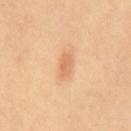Q: Is there a histopathology result?
A: total-body-photography surveillance lesion; no biopsy
Q: What lighting was used for the tile?
A: cross-polarized illumination
Q: What kind of image is this?
A: 15 mm crop, total-body photography
Q: What are the patient's age and sex?
A: female, aged 48–52
Q: How large is the lesion?
A: ≈3 mm